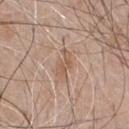This lesion was catalogued during total-body skin photography and was not selected for biopsy. A male subject, about 65 years old. From the front of the torso. Imaged with white-light lighting. A lesion tile, about 15 mm wide, cut from a 3D total-body photograph. The recorded lesion diameter is about 3.5 mm.